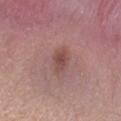The lesion was tiled from a total-body skin photograph and was not biopsied.
A female subject, aged 33 to 37.
A region of skin cropped from a whole-body photographic capture, roughly 15 mm wide.
Captured under white-light illumination.
The lesion is located on the right forearm.
Automated tile analysis of the lesion measured an eccentricity of roughly 0.65 and a shape-asymmetry score of about 0.2 (0 = symmetric). It also reported an average lesion color of about L≈48 a*≈22 b*≈22 (CIELAB) and a normalized lesion–skin contrast near 6.5. And it measured border irregularity of about 2 on a 0–10 scale and a peripheral color-asymmetry measure near 0.5. And it measured a nevus-likeness score of about 30/100 and a lesion-detection confidence of about 100/100.
The lesion's longest dimension is about 2.5 mm.